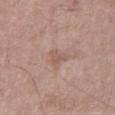Impression:
Recorded during total-body skin imaging; not selected for excision or biopsy.
Context:
A female subject about 75 years old. The tile uses white-light illumination. The lesion's longest dimension is about 2.5 mm. On the left thigh. Cropped from a whole-body photographic skin survey; the tile spans about 15 mm.